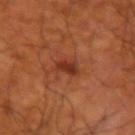notes: no biopsy performed (imaged during a skin exam) | lesion size: about 3 mm | patient: male, about 65 years old | image-analysis metrics: a lesion area of about 4 mm², an outline eccentricity of about 0.85 (0 = round, 1 = elongated), and a shape-asymmetry score of about 0.35 (0 = symmetric); a lesion color around L≈36 a*≈29 b*≈33 in CIELAB, a lesion–skin lightness drop of about 10, and a normalized border contrast of about 8.5 | imaging modality: ~15 mm crop, total-body skin-cancer survey | location: the right upper arm.